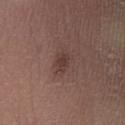workup: imaged on a skin check; not biopsied
location: the right lower leg
TBP lesion metrics: a lesion color around L≈37 a*≈17 b*≈20 in CIELAB and a lesion–skin lightness drop of about 8; internal color variation of about 1.5 on a 0–10 scale and radial color variation of about 0.5
patient: female, in their mid- to late 30s
size: about 2.5 mm
acquisition: total-body-photography crop, ~15 mm field of view